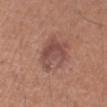workup = total-body-photography surveillance lesion; no biopsy | diameter = about 5 mm | imaging modality = ~15 mm tile from a whole-body skin photo | location = the right upper arm | patient = male, aged 53 to 57 | automated lesion analysis = an average lesion color of about L≈48 a*≈22 b*≈24 (CIELAB) and a lesion–skin lightness drop of about 9; a border-irregularity index near 6/10 and a peripheral color-asymmetry measure near 1; a nevus-likeness score of about 35/100 and a lesion-detection confidence of about 100/100.